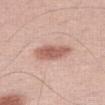  biopsy_status: not biopsied; imaged during a skin examination
  automated_metrics:
    cielab_L: 59
    cielab_a: 23
    cielab_b: 27
    vs_skin_darker_L: 13.0
    vs_skin_contrast_norm: 8.0
    border_irregularity_0_10: 2.5
    color_variation_0_10: 2.5
    peripheral_color_asymmetry: 1.0
    nevus_likeness_0_100: 85
  lighting: white-light
  site: leg
  patient:
    sex: male
    age_approx: 60
  image:
    source: total-body photography crop
    field_of_view_mm: 15
  lesion_size:
    long_diameter_mm_approx: 4.5